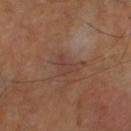The lesion was tiled from a total-body skin photograph and was not biopsied.
Cropped from a whole-body photographic skin survey; the tile spans about 15 mm.
From the leg.
A male patient, about 65 years old.
Automated tile analysis of the lesion measured a lesion area of about 4.5 mm² and a shape eccentricity near 0.75. The software also gave a lesion–skin lightness drop of about 5 and a normalized lesion–skin contrast near 4.5.